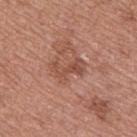notes = total-body-photography surveillance lesion; no biopsy | body site = the back | size = ~4 mm (longest diameter) | image source = ~15 mm crop, total-body skin-cancer survey | lighting = white-light | patient = male, aged around 55.